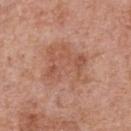workup: total-body-photography surveillance lesion; no biopsy | site: the chest | lighting: white-light | automated lesion analysis: an outline eccentricity of about 0.45 (0 = round, 1 = elongated) and two-axis asymmetry of about 0.35; about 7 CIELAB-L* units darker than the surrounding skin | image source: total-body-photography crop, ~15 mm field of view | diameter: ≈5.5 mm | subject: male, roughly 70 years of age.